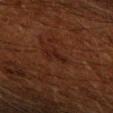notes: catalogued during a skin exam; not biopsied
tile lighting: cross-polarized
acquisition: ~15 mm crop, total-body skin-cancer survey
lesion diameter: about 2.5 mm
patient: male, in their 60s
anatomic site: the right upper arm
automated lesion analysis: a border-irregularity index near 5/10, internal color variation of about 0 on a 0–10 scale, and a peripheral color-asymmetry measure near 0